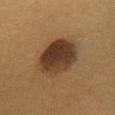This lesion was catalogued during total-body skin photography and was not selected for biopsy. The patient is a female in their 30s. A close-up tile cropped from a whole-body skin photograph, about 15 mm across. On the chest.